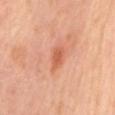– follow-up: no biopsy performed (imaged during a skin exam)
– image: ~15 mm tile from a whole-body skin photo
– site: the mid back
– subject: female, in their 50s
– lesion diameter: ~3 mm (longest diameter)
– image-analysis metrics: a lesion-detection confidence of about 100/100
– tile lighting: cross-polarized illumination